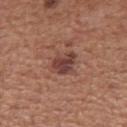Assessment:
Recorded during total-body skin imaging; not selected for excision or biopsy.
Acquisition and patient details:
Located on the chest. The tile uses white-light illumination. Automated tile analysis of the lesion measured an area of roughly 7 mm², a shape eccentricity near 0.6, and a symmetry-axis asymmetry near 0.2. And it measured a lesion–skin lightness drop of about 10. And it measured border irregularity of about 2 on a 0–10 scale and a within-lesion color-variation index near 4.5/10. A male patient aged 43–47. Cropped from a total-body skin-imaging series; the visible field is about 15 mm.